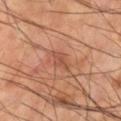Captured during whole-body skin photography for melanoma surveillance; the lesion was not biopsied. A male patient aged 48–52. A close-up tile cropped from a whole-body skin photograph, about 15 mm across. From the right lower leg.